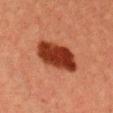This lesion was catalogued during total-body skin photography and was not selected for biopsy. The lesion is located on the chest. This image is a 15 mm lesion crop taken from a total-body photograph. The total-body-photography lesion software estimated an average lesion color of about L≈31 a*≈28 b*≈31 (CIELAB), a lesion–skin lightness drop of about 16, and a normalized lesion–skin contrast near 14. The software also gave an automated nevus-likeness rating near 100 out of 100 and a detector confidence of about 100 out of 100 that the crop contains a lesion. Imaged with cross-polarized lighting. The patient is a male roughly 40 years of age. The recorded lesion diameter is about 6 mm.